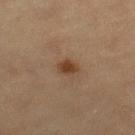{
  "biopsy_status": "not biopsied; imaged during a skin examination",
  "site": "right lower leg",
  "image": {
    "source": "total-body photography crop",
    "field_of_view_mm": 15
  },
  "patient": {
    "sex": "female",
    "age_approx": 65
  },
  "lighting": "cross-polarized"
}